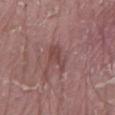<tbp_lesion>
<biopsy_status>not biopsied; imaged during a skin examination</biopsy_status>
<image>
  <source>total-body photography crop</source>
  <field_of_view_mm>15</field_of_view_mm>
</image>
<site>mid back</site>
<patient>
  <sex>male</sex>
  <age_approx>40</age_approx>
</patient>
</tbp_lesion>